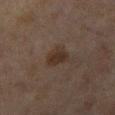notes=no biopsy performed (imaged during a skin exam)
body site=the right lower leg
tile lighting=cross-polarized
subject=female, aged 58 to 62
acquisition=~15 mm tile from a whole-body skin photo